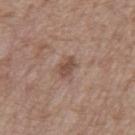Q: Was a biopsy performed?
A: total-body-photography surveillance lesion; no biopsy
Q: Who is the patient?
A: male, roughly 70 years of age
Q: What is the imaging modality?
A: ~15 mm crop, total-body skin-cancer survey
Q: What is the lesion's diameter?
A: ~2.5 mm (longest diameter)
Q: Where on the body is the lesion?
A: the back